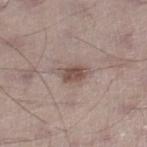Image and clinical context: A 15 mm close-up tile from a total-body photography series done for melanoma screening. Captured under white-light illumination. The patient is a male in their 70s. Automated image analysis of the tile measured an automated nevus-likeness rating near 80 out of 100. On the right lower leg. Approximately 3.5 mm at its widest.